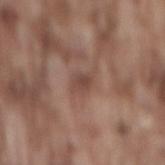| key | value |
|---|---|
| lesion diameter | ~3 mm (longest diameter) |
| tile lighting | white-light illumination |
| acquisition | total-body-photography crop, ~15 mm field of view |
| location | the mid back |
| patient | male, aged around 75 |